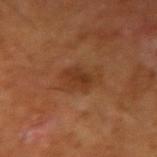Background: A male patient in their 60s. Imaged with cross-polarized lighting. Located on the right upper arm. About 4 mm across. A region of skin cropped from a whole-body photographic capture, roughly 15 mm wide. Automated image analysis of the tile measured a shape eccentricity near 0.8 and a shape-asymmetry score of about 0.35 (0 = symmetric). It also reported border irregularity of about 4 on a 0–10 scale, a within-lesion color-variation index near 3/10, and a peripheral color-asymmetry measure near 1. The analysis additionally found a classifier nevus-likeness of about 60/100.